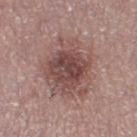Impression:
No biopsy was performed on this lesion — it was imaged during a full skin examination and was not determined to be concerning.